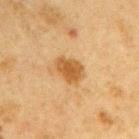follow-up: catalogued during a skin exam; not biopsied
acquisition: ~15 mm crop, total-body skin-cancer survey
lighting: cross-polarized illumination
lesion size: about 3.5 mm
location: the left upper arm
patient: male, aged 63–67
automated metrics: a footprint of about 6.5 mm², an outline eccentricity of about 0.7 (0 = round, 1 = elongated), and two-axis asymmetry of about 0.25; a mean CIELAB color near L≈45 a*≈19 b*≈37 and a lesion–skin lightness drop of about 10; a border-irregularity rating of about 2/10 and peripheral color asymmetry of about 1